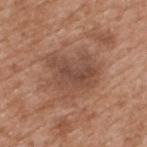* notes · no biopsy performed (imaged during a skin exam)
* location · the upper back
* subject · male, aged 63–67
* imaging modality · ~15 mm crop, total-body skin-cancer survey
* automated metrics · a lesion color around L≈48 a*≈20 b*≈28 in CIELAB, a lesion–skin lightness drop of about 8, and a lesion-to-skin contrast of about 6.5 (normalized; higher = more distinct); a within-lesion color-variation index near 4/10 and radial color variation of about 1.5
* lesion size · ≈5.5 mm